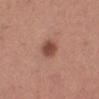follow-up: imaged on a skin check; not biopsied | image source: ~15 mm tile from a whole-body skin photo | lighting: white-light | patient: female, aged approximately 40 | lesion size: about 2.5 mm | image-analysis metrics: an area of roughly 5 mm², an outline eccentricity of about 0.6 (0 = round, 1 = elongated), and two-axis asymmetry of about 0.15 | location: the leg.